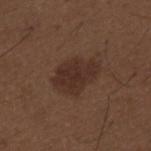follow-up: total-body-photography surveillance lesion; no biopsy | lighting: white-light | diameter: ≈5.5 mm | body site: the back | acquisition: ~15 mm tile from a whole-body skin photo | automated lesion analysis: an area of roughly 14 mm², an eccentricity of roughly 0.75, and a symmetry-axis asymmetry near 0.2; a mean CIELAB color near L≈30 a*≈17 b*≈22, roughly 7 lightness units darker than nearby skin, and a normalized border contrast of about 7.5; border irregularity of about 2.5 on a 0–10 scale and peripheral color asymmetry of about 1; an automated nevus-likeness rating near 45 out of 100 and a detector confidence of about 100 out of 100 that the crop contains a lesion | patient: male, aged approximately 50.